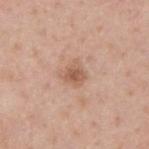workup: imaged on a skin check; not biopsied
acquisition: ~15 mm crop, total-body skin-cancer survey
patient: male, aged approximately 40
location: the upper back